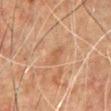Captured during whole-body skin photography for melanoma surveillance; the lesion was not biopsied.
The subject is a male aged around 50.
From the chest.
The tile uses cross-polarized illumination.
Automated tile analysis of the lesion measured a lesion–skin lightness drop of about 6 and a normalized lesion–skin contrast near 5. The software also gave a border-irregularity index near 3/10 and peripheral color asymmetry of about 0.5.
A 15 mm close-up extracted from a 3D total-body photography capture.
The recorded lesion diameter is about 3 mm.